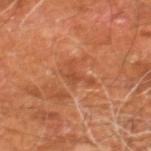workup: imaged on a skin check; not biopsied
patient: male, aged around 60
size: ~3.5 mm (longest diameter)
image source: ~15 mm crop, total-body skin-cancer survey
anatomic site: the right leg